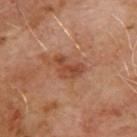  automated_metrics:
    area_mm2_approx: 6.0
    eccentricity: 0.8
    shape_asymmetry: 0.55
    nevus_likeness_0_100: 0
    lesion_detection_confidence_0_100: 100
  site: upper back
  image:
    source: total-body photography crop
    field_of_view_mm: 15
  patient:
    sex: male
    age_approx: 65